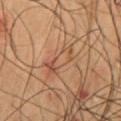Clinical impression: Recorded during total-body skin imaging; not selected for excision or biopsy. Context: The patient is a male aged 48–52. Imaged with cross-polarized lighting. A lesion tile, about 15 mm wide, cut from a 3D total-body photograph. Located on the mid back. The recorded lesion diameter is about 4 mm.